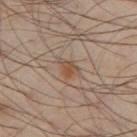workup — no biopsy performed (imaged during a skin exam); lighting — cross-polarized; size — ~3 mm (longest diameter); location — the left thigh; patient — male, in their 40s; automated metrics — a nevus-likeness score of about 60/100 and lesion-presence confidence of about 100/100; acquisition — 15 mm crop, total-body photography.